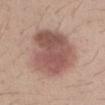notes: total-body-photography surveillance lesion; no biopsy
patient: male, aged 28–32
body site: the left forearm
automated metrics: a lesion area of about 32 mm²; a border-irregularity rating of about 2.5/10 and internal color variation of about 5 on a 0–10 scale
imaging modality: 15 mm crop, total-body photography
size: about 7.5 mm